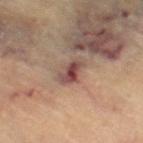notes=imaged on a skin check; not biopsied
subject=female, about 65 years old
acquisition=15 mm crop, total-body photography
anatomic site=the left leg